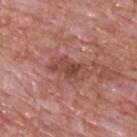Recorded during total-body skin imaging; not selected for excision or biopsy.
A male patient, in their 60s.
Cropped from a whole-body photographic skin survey; the tile spans about 15 mm.
Automated image analysis of the tile measured an area of roughly 7.5 mm², a shape eccentricity near 0.85, and a symmetry-axis asymmetry near 0.25. And it measured a color-variation rating of about 5/10 and radial color variation of about 2. It also reported a detector confidence of about 100 out of 100 that the crop contains a lesion.
Located on the upper back.